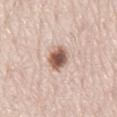| field | value |
|---|---|
| biopsy status | catalogued during a skin exam; not biopsied |
| lesion size | ~3.5 mm (longest diameter) |
| anatomic site | the mid back |
| illumination | white-light |
| subject | male, aged 78–82 |
| image-analysis metrics | a border-irregularity rating of about 1.5/10 and radial color variation of about 2; a classifier nevus-likeness of about 100/100 and lesion-presence confidence of about 100/100 |
| imaging modality | total-body-photography crop, ~15 mm field of view |